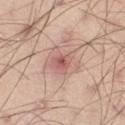Recorded during total-body skin imaging; not selected for excision or biopsy. A male patient in their mid-40s. The lesion's longest dimension is about 4.5 mm. The lesion is on the right thigh. A lesion tile, about 15 mm wide, cut from a 3D total-body photograph.